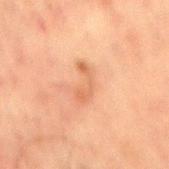workup: total-body-photography surveillance lesion; no biopsy
subject: male, aged approximately 50
acquisition: total-body-photography crop, ~15 mm field of view
anatomic site: the mid back